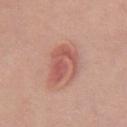The lesion was tiled from a total-body skin photograph and was not biopsied.
A lesion tile, about 15 mm wide, cut from a 3D total-body photograph.
The lesion's longest dimension is about 4 mm.
The subject is a female aged around 40.
Located on the chest.